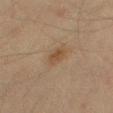Q: Was a biopsy performed?
A: imaged on a skin check; not biopsied
Q: What kind of image is this?
A: 15 mm crop, total-body photography
Q: Where on the body is the lesion?
A: the right thigh
Q: Illumination type?
A: cross-polarized
Q: What did automated image analysis measure?
A: an area of roughly 4 mm², an outline eccentricity of about 0.8 (0 = round, 1 = elongated), and a symmetry-axis asymmetry near 0.2; an average lesion color of about L≈39 a*≈14 b*≈27 (CIELAB) and a normalized border contrast of about 6.5; a border-irregularity rating of about 2/10, internal color variation of about 2 on a 0–10 scale, and radial color variation of about 0.5
Q: What is the lesion's diameter?
A: ≈3 mm
Q: Patient demographics?
A: male, aged around 40